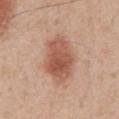follow-up: total-body-photography surveillance lesion; no biopsy | image: ~15 mm tile from a whole-body skin photo | tile lighting: white-light illumination | subject: male, aged around 55 | automated metrics: an area of roughly 17 mm², an outline eccentricity of about 0.75 (0 = round, 1 = elongated), and a shape-asymmetry score of about 0.2 (0 = symmetric); a lesion color around L≈55 a*≈23 b*≈30 in CIELAB, about 12 CIELAB-L* units darker than the surrounding skin, and a lesion-to-skin contrast of about 8.5 (normalized; higher = more distinct) | location: the abdomen.